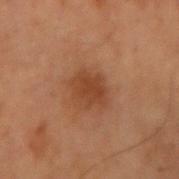Part of a total-body skin-imaging series; this lesion was reviewed on a skin check and was not flagged for biopsy. The lesion is located on the right upper arm. A close-up tile cropped from a whole-body skin photograph, about 15 mm across. Longest diameter approximately 3.5 mm. The patient is a male aged 68 to 72. The tile uses cross-polarized illumination.